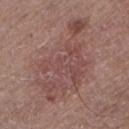The lesion is located on the left lower leg.
A 15 mm close-up extracted from a 3D total-body photography capture.
A male subject, aged approximately 65.